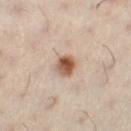{"biopsy_status": "not biopsied; imaged during a skin examination", "lighting": "cross-polarized", "automated_metrics": {"area_mm2_approx": 5.5, "shape_asymmetry": 0.2, "cielab_L": 43, "cielab_a": 16, "cielab_b": 25, "vs_skin_contrast_norm": 10.5}, "patient": {"sex": "female", "age_approx": 30}, "site": "left lower leg", "image": {"source": "total-body photography crop", "field_of_view_mm": 15}}